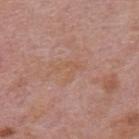<case>
<biopsy_status>not biopsied; imaged during a skin examination</biopsy_status>
<site>upper back</site>
<lighting>white-light</lighting>
<lesion_size>
  <long_diameter_mm_approx>3.5</long_diameter_mm_approx>
</lesion_size>
<image>
  <source>total-body photography crop</source>
  <field_of_view_mm>15</field_of_view_mm>
</image>
<automated_metrics>
  <area_mm2_approx>4.0</area_mm2_approx>
  <eccentricity>0.8</eccentricity>
  <shape_asymmetry>0.55</shape_asymmetry>
  <color_variation_0_10>0.0</color_variation_0_10>
  <peripheral_color_asymmetry>0.0</peripheral_color_asymmetry>
  <nevus_likeness_0_100>0</nevus_likeness_0_100>
</automated_metrics>
<patient>
  <sex>male</sex>
  <age_approx>75</age_approx>
</patient>
</case>